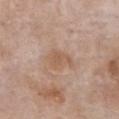Acquisition and patient details: Located on the chest. Automated tile analysis of the lesion measured a lesion area of about 5 mm², an eccentricity of roughly 0.75, and a shape-asymmetry score of about 0.45 (0 = symmetric). It also reported an average lesion color of about L≈57 a*≈18 b*≈30 (CIELAB), a lesion–skin lightness drop of about 7, and a normalized border contrast of about 5.5. The analysis additionally found a border-irregularity rating of about 4/10, a within-lesion color-variation index near 2/10, and radial color variation of about 1. The analysis additionally found an automated nevus-likeness rating near 0 out of 100 and a detector confidence of about 100 out of 100 that the crop contains a lesion. A female subject aged approximately 85 or older. This is a white-light tile. This image is a 15 mm lesion crop taken from a total-body photograph. Measured at roughly 3 mm in maximum diameter.